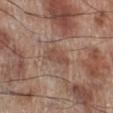Captured during whole-body skin photography for melanoma surveillance; the lesion was not biopsied. The subject is a male aged 68–72. A 15 mm close-up extracted from a 3D total-body photography capture. Captured under white-light illumination. From the right lower leg.